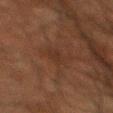follow-up = imaged on a skin check; not biopsied
subject = male, aged approximately 60
size = about 2.5 mm
tile lighting = cross-polarized illumination
image source = ~15 mm tile from a whole-body skin photo
site = the mid back